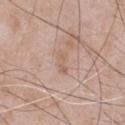Notes:
• follow-up: total-body-photography surveillance lesion; no biopsy
• acquisition: 15 mm crop, total-body photography
• body site: the chest
• patient: male, aged approximately 50